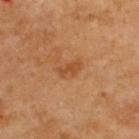Imaged during a routine full-body skin examination; the lesion was not biopsied and no histopathology is available. Imaged with cross-polarized lighting. The recorded lesion diameter is about 3 mm. A lesion tile, about 15 mm wide, cut from a 3D total-body photograph. Located on the back. The total-body-photography lesion software estimated an average lesion color of about L≈48 a*≈24 b*≈39 (CIELAB), a lesion–skin lightness drop of about 8, and a normalized lesion–skin contrast near 6. And it measured border irregularity of about 3.5 on a 0–10 scale and radial color variation of about 0. A male subject, roughly 70 years of age.